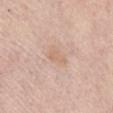{
  "biopsy_status": "not biopsied; imaged during a skin examination",
  "lesion_size": {
    "long_diameter_mm_approx": 3.0
  },
  "patient": {
    "sex": "female",
    "age_approx": 70
  },
  "image": {
    "source": "total-body photography crop",
    "field_of_view_mm": 15
  },
  "site": "chest",
  "lighting": "white-light"
}